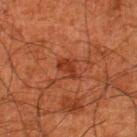Recorded during total-body skin imaging; not selected for excision or biopsy. The lesion is on the left lower leg. A 15 mm close-up tile from a total-body photography series done for melanoma screening. A male subject aged 78–82.